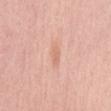biopsy status = catalogued during a skin exam; not biopsied | site = the mid back | image = ~15 mm crop, total-body skin-cancer survey | patient = female, approximately 60 years of age.